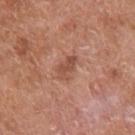Recorded during total-body skin imaging; not selected for excision or biopsy.
A 15 mm close-up tile from a total-body photography series done for melanoma screening.
About 3 mm across.
The lesion is on the right upper arm.
The lesion-visualizer software estimated an eccentricity of roughly 0.85 and a shape-asymmetry score of about 0.4 (0 = symmetric).
The tile uses white-light illumination.
The patient is a male about 65 years old.